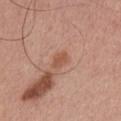The lesion was photographed on a routine skin check and not biopsied; there is no pathology result. Cropped from a whole-body photographic skin survey; the tile spans about 15 mm. Located on the chest. The tile uses white-light illumination. The lesion's longest dimension is about 2.5 mm. The patient is a male aged 28 to 32.